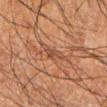* notes: catalogued during a skin exam; not biopsied
* lighting: cross-polarized
* patient: male, roughly 60 years of age
* image: ~15 mm crop, total-body skin-cancer survey
* location: the right forearm
* TBP lesion metrics: an area of roughly 3.5 mm², a shape eccentricity near 0.75, and a symmetry-axis asymmetry near 0.4; an average lesion color of about L≈37 a*≈18 b*≈26 (CIELAB) and a normalized border contrast of about 5.5; a border-irregularity rating of about 4/10, a within-lesion color-variation index near 1.5/10, and a peripheral color-asymmetry measure near 0.5; an automated nevus-likeness rating near 0 out of 100 and lesion-presence confidence of about 70/100